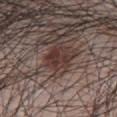Clinical impression:
Recorded during total-body skin imaging; not selected for excision or biopsy.
Background:
The lesion is located on the abdomen. About 3.5 mm across. A region of skin cropped from a whole-body photographic capture, roughly 15 mm wide. Automated tile analysis of the lesion measured a mean CIELAB color near L≈34 a*≈17 b*≈19 and a lesion-to-skin contrast of about 8.5 (normalized; higher = more distinct). The software also gave border irregularity of about 2.5 on a 0–10 scale, a color-variation rating of about 4.5/10, and radial color variation of about 1.5. The software also gave an automated nevus-likeness rating near 95 out of 100 and lesion-presence confidence of about 100/100. A male subject, aged 68–72.